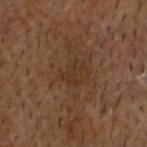The lesion was photographed on a routine skin check and not biopsied; there is no pathology result.
A male patient, aged around 60.
A region of skin cropped from a whole-body photographic capture, roughly 15 mm wide.
On the head or neck.
About 3 mm across.